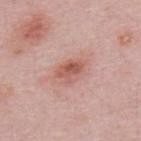notes: total-body-photography surveillance lesion; no biopsy
anatomic site: the upper back
patient: male, roughly 50 years of age
imaging modality: ~15 mm tile from a whole-body skin photo